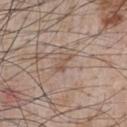biopsy status = imaged on a skin check; not biopsied | patient = male, in their mid- to late 60s | diameter = about 3 mm | acquisition = total-body-photography crop, ~15 mm field of view | anatomic site = the front of the torso.